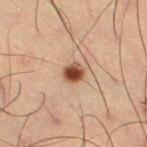{"biopsy_status": "not biopsied; imaged during a skin examination", "image": {"source": "total-body photography crop", "field_of_view_mm": 15}, "site": "left thigh", "lighting": "cross-polarized", "lesion_size": {"long_diameter_mm_approx": 2.5}, "patient": {"sex": "male", "age_approx": 55}}